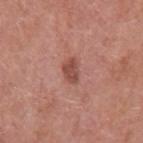automated lesion analysis = an average lesion color of about L≈49 a*≈25 b*≈26 (CIELAB), about 11 CIELAB-L* units darker than the surrounding skin, and a normalized border contrast of about 7.5; a nevus-likeness score of about 40/100 and lesion-presence confidence of about 100/100
diameter = ≈2.5 mm
site = the left upper arm
image = ~15 mm tile from a whole-body skin photo
illumination = white-light
patient = male, roughly 70 years of age